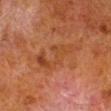No biopsy was performed on this lesion — it was imaged during a full skin examination and was not determined to be concerning. Cropped from a whole-body photographic skin survey; the tile spans about 15 mm. Measured at roughly 7 mm in maximum diameter. The subject is a male approximately 80 years of age. The lesion is located on the right lower leg.